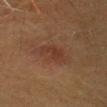Impression: Recorded during total-body skin imaging; not selected for excision or biopsy. Image and clinical context: The lesion's longest dimension is about 4 mm. A female subject, approximately 40 years of age. Imaged with cross-polarized lighting. Cropped from a whole-body photographic skin survey; the tile spans about 15 mm. From the head or neck.